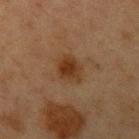Recorded during total-body skin imaging; not selected for excision or biopsy.
An algorithmic analysis of the crop reported an eccentricity of roughly 0.6 and two-axis asymmetry of about 0.25. It also reported border irregularity of about 2 on a 0–10 scale, a within-lesion color-variation index near 3/10, and a peripheral color-asymmetry measure near 1. And it measured an automated nevus-likeness rating near 95 out of 100 and a detector confidence of about 100 out of 100 that the crop contains a lesion.
The subject is a female about 40 years old.
Cropped from a total-body skin-imaging series; the visible field is about 15 mm.
The tile uses cross-polarized illumination.
Measured at roughly 3.5 mm in maximum diameter.
On the left upper arm.